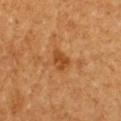notes = total-body-photography surveillance lesion; no biopsy
TBP lesion metrics = a border-irregularity rating of about 2.5/10, a color-variation rating of about 2.5/10, and a peripheral color-asymmetry measure near 1; a classifier nevus-likeness of about 50/100 and a lesion-detection confidence of about 100/100
image = ~15 mm tile from a whole-body skin photo
lighting = cross-polarized
lesion size = ~2.5 mm (longest diameter)
patient = female, aged approximately 30
location = the chest